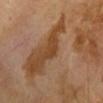<case>
<biopsy_status>not biopsied; imaged during a skin examination</biopsy_status>
<lesion_size>
  <long_diameter_mm_approx>2.5</long_diameter_mm_approx>
</lesion_size>
<site>right forearm</site>
<image>
  <source>total-body photography crop</source>
  <field_of_view_mm>15</field_of_view_mm>
</image>
<lighting>cross-polarized</lighting>
<patient>
  <sex>female</sex>
  <age_approx>40</age_approx>
</patient>
</case>